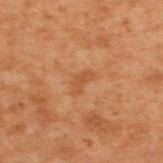biopsy status = imaged on a skin check; not biopsied
subject = male, roughly 70 years of age
anatomic site = the upper back
diameter = about 2.5 mm
image source = 15 mm crop, total-body photography
image-analysis metrics = an average lesion color of about L≈39 a*≈20 b*≈32 (CIELAB), a lesion–skin lightness drop of about 5, and a normalized border contrast of about 5; a within-lesion color-variation index near 0.5/10; an automated nevus-likeness rating near 0 out of 100 and a lesion-detection confidence of about 100/100